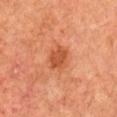biopsy_status: not biopsied; imaged during a skin examination
automated_metrics:
  cielab_L: 47
  cielab_a: 29
  cielab_b: 36
  vs_skin_darker_L: 9.0
image:
  source: total-body photography crop
  field_of_view_mm: 15
lesion_size:
  long_diameter_mm_approx: 3.0
site: right upper arm
patient:
  sex: male
  age_approx: 65
lighting: cross-polarized